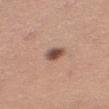workup: total-body-photography surveillance lesion; no biopsy
lesion diameter: ≈2.5 mm
subject: female, aged 28–32
anatomic site: the leg
imaging modality: ~15 mm crop, total-body skin-cancer survey